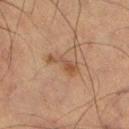Recorded during total-body skin imaging; not selected for excision or biopsy.